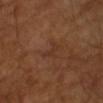This lesion was catalogued during total-body skin photography and was not selected for biopsy.
Automated image analysis of the tile measured an average lesion color of about L≈32 a*≈20 b*≈27 (CIELAB), about 5 CIELAB-L* units darker than the surrounding skin, and a normalized lesion–skin contrast near 4.5.
A male patient about 65 years old.
Captured under cross-polarized illumination.
A 15 mm crop from a total-body photograph taken for skin-cancer surveillance.
Longest diameter approximately 2.5 mm.